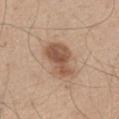| key | value |
|---|---|
| biopsy status | catalogued during a skin exam; not biopsied |
| lighting | white-light illumination |
| image | ~15 mm tile from a whole-body skin photo |
| size | ≈4.5 mm |
| patient | male, in their 60s |
| location | the left thigh |